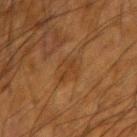| key | value |
|---|---|
| workup | total-body-photography surveillance lesion; no biopsy |
| subject | male, about 65 years old |
| body site | the left forearm |
| lesion size | ≈3.5 mm |
| tile lighting | cross-polarized |
| imaging modality | 15 mm crop, total-body photography |
| TBP lesion metrics | an area of roughly 5.5 mm², an outline eccentricity of about 0.75 (0 = round, 1 = elongated), and a shape-asymmetry score of about 0.35 (0 = symmetric); an average lesion color of about L≈31 a*≈17 b*≈31 (CIELAB) and about 4 CIELAB-L* units darker than the surrounding skin; a within-lesion color-variation index near 2/10 |